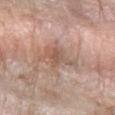  site: left forearm
  patient:
    sex: female
    age_approx: 65
  lighting: white-light
  image:
    source: total-body photography crop
    field_of_view_mm: 15